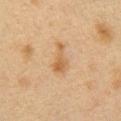<tbp_lesion>
<biopsy_status>not biopsied; imaged during a skin examination</biopsy_status>
<patient>
  <sex>female</sex>
  <age_approx>40</age_approx>
</patient>
<lesion_size>
  <long_diameter_mm_approx>4.5</long_diameter_mm_approx>
</lesion_size>
<image>
  <source>total-body photography crop</source>
  <field_of_view_mm>15</field_of_view_mm>
</image>
<site>chest</site>
<lighting>cross-polarized</lighting>
</tbp_lesion>